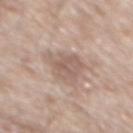Notes:
• notes · imaged on a skin check; not biopsied
• patient · male, aged 78–82
• lesion size · ≈4 mm
• site · the back
• TBP lesion metrics · a shape-asymmetry score of about 0.4 (0 = symmetric); border irregularity of about 5 on a 0–10 scale, a color-variation rating of about 2.5/10, and peripheral color asymmetry of about 1
• imaging modality · ~15 mm crop, total-body skin-cancer survey
• lighting · white-light illumination